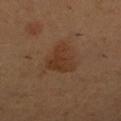Captured during whole-body skin photography for melanoma surveillance; the lesion was not biopsied. The recorded lesion diameter is about 4 mm. The lesion-visualizer software estimated an average lesion color of about L≈34 a*≈18 b*≈29 (CIELAB). The software also gave a border-irregularity index near 4.5/10 and a within-lesion color-variation index near 2.5/10. The software also gave a classifier nevus-likeness of about 70/100 and a detector confidence of about 100 out of 100 that the crop contains a lesion. The lesion is on the left leg. A female patient, approximately 45 years of age. A 15 mm close-up tile from a total-body photography series done for melanoma screening.